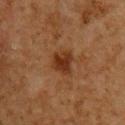Clinical impression:
Part of a total-body skin-imaging series; this lesion was reviewed on a skin check and was not flagged for biopsy.
Image and clinical context:
About 3 mm across. A male subject aged 58–62. On the chest. A roughly 15 mm field-of-view crop from a total-body skin photograph.